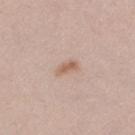{"biopsy_status": "not biopsied; imaged during a skin examination", "patient": {"sex": "female", "age_approx": 40}, "image": {"source": "total-body photography crop", "field_of_view_mm": 15}, "site": "leg", "automated_metrics": {"border_irregularity_0_10": 3.0, "color_variation_0_10": 0.5, "peripheral_color_asymmetry": 0.0}, "lighting": "white-light", "lesion_size": {"long_diameter_mm_approx": 2.5}}